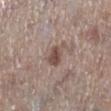| feature | finding |
|---|---|
| notes | imaged on a skin check; not biopsied |
| body site | the left lower leg |
| patient | female, aged approximately 65 |
| imaging modality | total-body-photography crop, ~15 mm field of view |